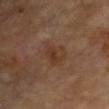{
  "lesion_size": {
    "long_diameter_mm_approx": 3.5
  },
  "lighting": "cross-polarized",
  "site": "chest",
  "patient": {
    "sex": "female",
    "age_approx": 70
  },
  "image": {
    "source": "total-body photography crop",
    "field_of_view_mm": 15
  },
  "automated_metrics": {
    "cielab_L": 34,
    "cielab_a": 17,
    "cielab_b": 27,
    "vs_skin_darker_L": 6.0,
    "vs_skin_contrast_norm": 6.0
  }
}